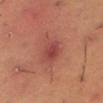Q: Was a biopsy performed?
A: no biopsy performed (imaged during a skin exam)
Q: Where on the body is the lesion?
A: the leg
Q: Lesion size?
A: ~3.5 mm (longest diameter)
Q: Illumination type?
A: cross-polarized
Q: What is the imaging modality?
A: ~15 mm crop, total-body skin-cancer survey
Q: Automated lesion metrics?
A: an average lesion color of about L≈45 a*≈30 b*≈25 (CIELAB); a border-irregularity rating of about 1.5/10, internal color variation of about 4 on a 0–10 scale, and radial color variation of about 1.5
Q: Who is the patient?
A: male, aged approximately 50